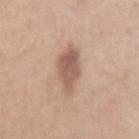No biopsy was performed on this lesion — it was imaged during a full skin examination and was not determined to be concerning. The lesion's longest dimension is about 6 mm. This image is a 15 mm lesion crop taken from a total-body photograph. The tile uses white-light illumination. The lesion is on the mid back. A male subject aged 28 to 32.